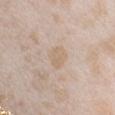Case summary:
• follow-up — no biopsy performed (imaged during a skin exam)
• lesion diameter — ~3 mm (longest diameter)
• image-analysis metrics — a footprint of about 5.5 mm², an outline eccentricity of about 0.65 (0 = round, 1 = elongated), and two-axis asymmetry of about 0.2; a nevus-likeness score of about 0/100 and lesion-presence confidence of about 100/100
• lighting — white-light
• patient — female, about 25 years old
• location — the chest
• acquisition — ~15 mm tile from a whole-body skin photo